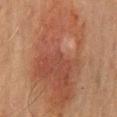Assessment: The lesion was photographed on a routine skin check and not biopsied; there is no pathology result. Acquisition and patient details: The tile uses cross-polarized illumination. The lesion is located on the abdomen. Cropped from a whole-body photographic skin survey; the tile spans about 15 mm. A male patient in their mid-60s.